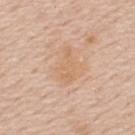workup: total-body-photography surveillance lesion; no biopsy | subject: male, aged around 60 | body site: the upper back | imaging modality: total-body-photography crop, ~15 mm field of view.